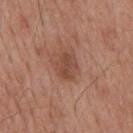Q: Was this lesion biopsied?
A: no biopsy performed (imaged during a skin exam)
Q: Who is the patient?
A: male, aged 53 to 57
Q: What is the anatomic site?
A: the mid back
Q: How was this image acquired?
A: total-body-photography crop, ~15 mm field of view